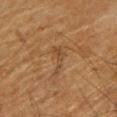Assessment:
Part of a total-body skin-imaging series; this lesion was reviewed on a skin check and was not flagged for biopsy.
Acquisition and patient details:
Located on the left lower leg. A 15 mm close-up extracted from a 3D total-body photography capture. A male subject, aged approximately 60.